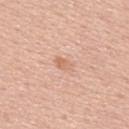{"biopsy_status": "not biopsied; imaged during a skin examination", "image": {"source": "total-body photography crop", "field_of_view_mm": 15}, "patient": {"sex": "male", "age_approx": 65}, "site": "upper back", "lighting": "white-light"}